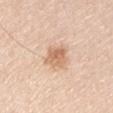workup: no biopsy performed (imaged during a skin exam)
site: the arm
tile lighting: white-light illumination
image: ~15 mm crop, total-body skin-cancer survey
patient: male, roughly 45 years of age